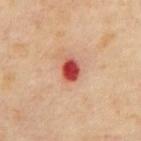Clinical impression:
Part of a total-body skin-imaging series; this lesion was reviewed on a skin check and was not flagged for biopsy.
Background:
Automated tile analysis of the lesion measured a footprint of about 5 mm² and an eccentricity of roughly 0.7. And it measured an average lesion color of about L≈49 a*≈37 b*≈31 (CIELAB) and about 18 CIELAB-L* units darker than the surrounding skin. The software also gave a border-irregularity rating of about 1/10. A male subject, roughly 65 years of age. Cropped from a whole-body photographic skin survey; the tile spans about 15 mm. The tile uses cross-polarized illumination.